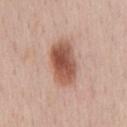Notes:
– tile lighting: white-light illumination
– automated lesion analysis: a shape eccentricity near 0.8 and a symmetry-axis asymmetry near 0.15; a nevus-likeness score of about 100/100 and lesion-presence confidence of about 100/100
– body site: the front of the torso
– image source: 15 mm crop, total-body photography
– subject: male, aged 53–57